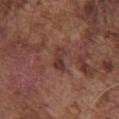Clinical impression:
The lesion was tiled from a total-body skin photograph and was not biopsied.
Clinical summary:
About 3 mm across. The patient is a male approximately 75 years of age. Automated tile analysis of the lesion measured a footprint of about 4 mm², an eccentricity of roughly 0.85, and a shape-asymmetry score of about 0.35 (0 = symmetric). It also reported a lesion color around L≈36 a*≈21 b*≈23 in CIELAB, roughly 8 lightness units darker than nearby skin, and a lesion-to-skin contrast of about 7 (normalized; higher = more distinct). The analysis additionally found a within-lesion color-variation index near 4/10 and a peripheral color-asymmetry measure near 1.5. From the chest. A 15 mm close-up tile from a total-body photography series done for melanoma screening.